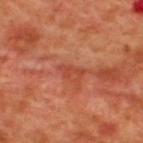workup: no biopsy performed (imaged during a skin exam)
imaging modality: ~15 mm crop, total-body skin-cancer survey
lighting: cross-polarized illumination
patient: male, aged approximately 50
automated metrics: a lesion area of about 2.5 mm², a shape eccentricity near 0.9, and two-axis asymmetry of about 0.5; a classifier nevus-likeness of about 0/100 and lesion-presence confidence of about 80/100
site: the upper back